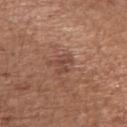Part of a total-body skin-imaging series; this lesion was reviewed on a skin check and was not flagged for biopsy.
The lesion's longest dimension is about 2.5 mm.
The lesion is located on the right upper arm.
A male subject, in their mid-60s.
A roughly 15 mm field-of-view crop from a total-body skin photograph.